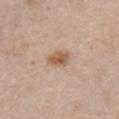workup: catalogued during a skin exam; not biopsied
location: the chest
lesion diameter: ≈2.5 mm
image: total-body-photography crop, ~15 mm field of view
tile lighting: white-light illumination
subject: female, aged 48–52